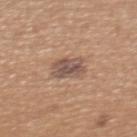site: back
lighting: white-light
patient:
  sex: female
  age_approx: 35
image:
  source: total-body photography crop
  field_of_view_mm: 15
automated_metrics:
  cielab_L: 52
  cielab_a: 17
  cielab_b: 25
  vs_skin_darker_L: 11.0
  vs_skin_contrast_norm: 8.5
  border_irregularity_0_10: 2.5
  color_variation_0_10: 4.5
  nevus_likeness_0_100: 15
  lesion_detection_confidence_0_100: 100